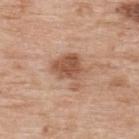<record>
  <biopsy_status>not biopsied; imaged during a skin examination</biopsy_status>
  <image>
    <source>total-body photography crop</source>
    <field_of_view_mm>15</field_of_view_mm>
  </image>
  <patient>
    <sex>male</sex>
    <age_approx>70</age_approx>
  </patient>
  <lesion_size>
    <long_diameter_mm_approx>4.5</long_diameter_mm_approx>
  </lesion_size>
  <lighting>white-light</lighting>
  <site>upper back</site>
</record>